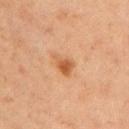Impression:
No biopsy was performed on this lesion — it was imaged during a full skin examination and was not determined to be concerning.
Clinical summary:
The patient is a female in their mid-40s. Cropped from a whole-body photographic skin survey; the tile spans about 15 mm. The lesion is on the left upper arm.